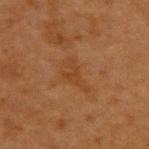Case summary:
– workup: catalogued during a skin exam; not biopsied
– patient: female, aged 38–42
– acquisition: 15 mm crop, total-body photography
– anatomic site: the upper back
– tile lighting: cross-polarized
– lesion diameter: ~4 mm (longest diameter)